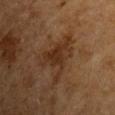biopsy status — catalogued during a skin exam; not biopsied | image source — ~15 mm crop, total-body skin-cancer survey | patient — male, approximately 65 years of age | location — the front of the torso | diameter — about 5.5 mm | tile lighting — cross-polarized | automated lesion analysis — a lesion color around L≈28 a*≈17 b*≈27 in CIELAB, a lesion–skin lightness drop of about 7, and a normalized border contrast of about 7.5.